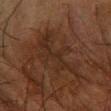Case summary:
* follow-up: catalogued during a skin exam; not biopsied
* image: ~15 mm crop, total-body skin-cancer survey
* subject: male, approximately 65 years of age
* site: the right forearm
* tile lighting: cross-polarized